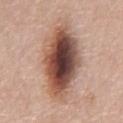notes — imaged on a skin check; not biopsied | patient — male, about 75 years old | body site — the abdomen | lesion diameter — ≈9 mm | image — 15 mm crop, total-body photography | illumination — white-light illumination | automated lesion analysis — a classifier nevus-likeness of about 100/100 and a detector confidence of about 100 out of 100 that the crop contains a lesion.